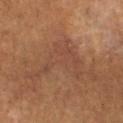Captured during whole-body skin photography for melanoma surveillance; the lesion was not biopsied. About 8 mm across. From the left lower leg. The lesion-visualizer software estimated an area of roughly 16 mm², an eccentricity of roughly 0.85, and a symmetry-axis asymmetry near 0.75. The software also gave a mean CIELAB color near L≈33 a*≈16 b*≈23. A female patient, about 80 years old. Captured under cross-polarized illumination. A lesion tile, about 15 mm wide, cut from a 3D total-body photograph.